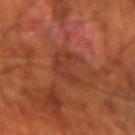image = ~15 mm crop, total-body skin-cancer survey | diameter = ~4 mm (longest diameter) | body site = the left forearm | subject = male, about 70 years old.